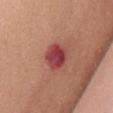Captured during whole-body skin photography for melanoma surveillance; the lesion was not biopsied.
This image is a 15 mm lesion crop taken from a total-body photograph.
The patient is a female aged 48–52.
The tile uses white-light illumination.
Automated tile analysis of the lesion measured a footprint of about 8 mm² and a shape eccentricity near 0.45. And it measured a lesion–skin lightness drop of about 14 and a lesion-to-skin contrast of about 10.5 (normalized; higher = more distinct).
Located on the front of the torso.
The recorded lesion diameter is about 3.5 mm.